A female patient approximately 60 years of age. Cropped from a whole-body photographic skin survey; the tile spans about 15 mm. Located on the upper back.
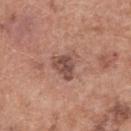{"lesion_size": {"long_diameter_mm_approx": 3.5}, "lighting": "white-light", "automated_metrics": {"area_mm2_approx": 7.0, "eccentricity": 0.4, "shape_asymmetry": 0.45, "cielab_L": 49, "cielab_a": 21, "cielab_b": 25, "vs_skin_contrast_norm": 8.0, "nevus_likeness_0_100": 10, "lesion_detection_confidence_0_100": 100}, "diagnosis": {"histopathology": "seborrheic keratosis", "malignancy": "benign", "taxonomic_path": ["Benign", "Benign epidermal proliferations", "Seborrheic keratosis"]}}